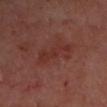biopsy status — imaged on a skin check; not biopsied | body site — the left forearm | imaging modality — ~15 mm crop, total-body skin-cancer survey | size — ≈4.5 mm | patient — female, aged around 55 | automated metrics — an automated nevus-likeness rating near 5 out of 100.